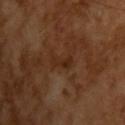workup = no biopsy performed (imaged during a skin exam)
imaging modality = 15 mm crop, total-body photography
automated metrics = about 5 CIELAB-L* units darker than the surrounding skin and a normalized border contrast of about 6; peripheral color asymmetry of about 0
diameter = about 3 mm
patient = male, aged 63–67
lighting = cross-polarized illumination